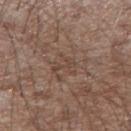Notes:
* follow-up · total-body-photography surveillance lesion; no biopsy
* imaging modality · ~15 mm tile from a whole-body skin photo
* body site · the right forearm
* patient · male, aged approximately 75
* lesion size · about 2.5 mm